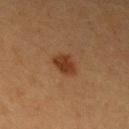Clinical impression: Captured during whole-body skin photography for melanoma surveillance; the lesion was not biopsied. Clinical summary: A female subject, about 40 years old. On the left upper arm. Imaged with cross-polarized lighting. About 3 mm across. A 15 mm crop from a total-body photograph taken for skin-cancer surveillance.